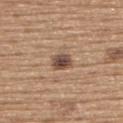Clinical impression:
The lesion was tiled from a total-body skin photograph and was not biopsied.
Context:
A roughly 15 mm field-of-view crop from a total-body skin photograph. The subject is a male in their mid- to late 60s. The lesion is on the upper back. Automated image analysis of the tile measured an outline eccentricity of about 0.6 (0 = round, 1 = elongated). The analysis additionally found a nevus-likeness score of about 80/100 and a detector confidence of about 100 out of 100 that the crop contains a lesion.